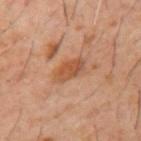Longest diameter approximately 4 mm. Cropped from a total-body skin-imaging series; the visible field is about 15 mm. Automated tile analysis of the lesion measured an outline eccentricity of about 0.85 (0 = round, 1 = elongated) and a symmetry-axis asymmetry near 0.2. And it measured a lesion color around L≈48 a*≈23 b*≈33 in CIELAB and a normalized border contrast of about 7. And it measured a nevus-likeness score of about 65/100. This is a cross-polarized tile. The patient is a male roughly 60 years of age. The lesion is on the mid back.